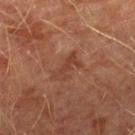workup: no biopsy performed (imaged during a skin exam)
acquisition: ~15 mm crop, total-body skin-cancer survey
subject: male, aged 73 to 77
lighting: cross-polarized
lesion size: ≈3.5 mm
body site: the leg